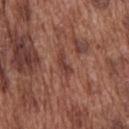<record>
<biopsy_status>not biopsied; imaged during a skin examination</biopsy_status>
<patient>
  <sex>male</sex>
  <age_approx>75</age_approx>
</patient>
<site>upper back</site>
<lighting>white-light</lighting>
<image>
  <source>total-body photography crop</source>
  <field_of_view_mm>15</field_of_view_mm>
</image>
<automated_metrics>
  <area_mm2_approx>3.5</area_mm2_approx>
  <eccentricity>0.9</eccentricity>
  <shape_asymmetry>0.4</shape_asymmetry>
  <border_irregularity_0_10>4.5</border_irregularity_0_10>
  <color_variation_0_10>0.5</color_variation_0_10>
</automated_metrics>
<lesion_size>
  <long_diameter_mm_approx>3.0</long_diameter_mm_approx>
</lesion_size>
</record>